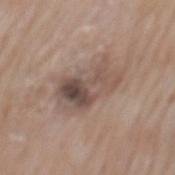notes=imaged on a skin check; not biopsied
location=the back
image source=~15 mm tile from a whole-body skin photo
subject=male, aged approximately 60